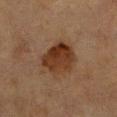Findings:
- workup — total-body-photography surveillance lesion; no biopsy
- anatomic site — the chest
- imaging modality — 15 mm crop, total-body photography
- tile lighting — cross-polarized illumination
- subject — female, aged 53 to 57
- lesion size — ~4.5 mm (longest diameter)